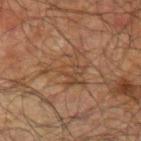Clinical impression: Part of a total-body skin-imaging series; this lesion was reviewed on a skin check and was not flagged for biopsy. Acquisition and patient details: The patient is a male about 70 years old. Measured at roughly 5 mm in maximum diameter. The tile uses cross-polarized illumination. From the left upper arm. A close-up tile cropped from a whole-body skin photograph, about 15 mm across.